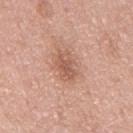Findings:
– follow-up — total-body-photography surveillance lesion; no biopsy
– diameter — about 4 mm
– subject — male, about 50 years old
– location — the mid back
– image — ~15 mm tile from a whole-body skin photo
– image-analysis metrics — a lesion area of about 7.5 mm², an outline eccentricity of about 0.85 (0 = round, 1 = elongated), and a symmetry-axis asymmetry near 0.2; a lesion color around L≈58 a*≈22 b*≈30 in CIELAB and a lesion–skin lightness drop of about 10; border irregularity of about 3 on a 0–10 scale, a within-lesion color-variation index near 3/10, and peripheral color asymmetry of about 1; lesion-presence confidence of about 100/100
– illumination — white-light illumination